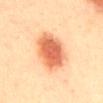Q: Was this lesion biopsied?
A: catalogued during a skin exam; not biopsied
Q: What did automated image analysis measure?
A: an area of roughly 19 mm², a shape eccentricity near 0.8, and a symmetry-axis asymmetry near 0.15; a lesion color around L≈61 a*≈27 b*≈37 in CIELAB; a border-irregularity rating of about 2/10, internal color variation of about 5.5 on a 0–10 scale, and a peripheral color-asymmetry measure near 1.5; a nevus-likeness score of about 100/100 and lesion-presence confidence of about 100/100
Q: What lighting was used for the tile?
A: cross-polarized
Q: Patient demographics?
A: male, aged 38–42
Q: Lesion location?
A: the mid back
Q: How was this image acquired?
A: 15 mm crop, total-body photography
Q: How large is the lesion?
A: ~6.5 mm (longest diameter)